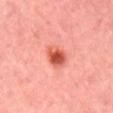No biopsy was performed on this lesion — it was imaged during a full skin examination and was not determined to be concerning.
The patient is a male roughly 45 years of age.
Measured at roughly 3 mm in maximum diameter.
A close-up tile cropped from a whole-body skin photograph, about 15 mm across.
The lesion is on the mid back.
Captured under cross-polarized illumination.